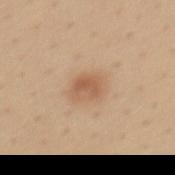| feature | finding |
|---|---|
| workup | imaged on a skin check; not biopsied |
| lighting | white-light illumination |
| size | ≈3 mm |
| automated lesion analysis | a lesion color around L≈58 a*≈20 b*≈33 in CIELAB and roughly 9 lightness units darker than nearby skin; a border-irregularity index near 3/10, a within-lesion color-variation index near 3.5/10, and peripheral color asymmetry of about 1 |
| image | ~15 mm crop, total-body skin-cancer survey |
| anatomic site | the mid back |
| subject | female, approximately 40 years of age |